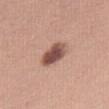Case summary:
• workup: imaged on a skin check; not biopsied
• subject: female, aged around 45
• location: the right thigh
• tile lighting: white-light illumination
• diameter: about 3.5 mm
• imaging modality: total-body-photography crop, ~15 mm field of view
• image-analysis metrics: a lesion area of about 7.5 mm², an outline eccentricity of about 0.7 (0 = round, 1 = elongated), and two-axis asymmetry of about 0.15; a mean CIELAB color near L≈51 a*≈21 b*≈25 and a lesion–skin lightness drop of about 16